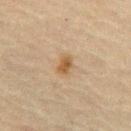Q: Is there a histopathology result?
A: imaged on a skin check; not biopsied
Q: Lesion size?
A: ~3 mm (longest diameter)
Q: How was the tile lit?
A: cross-polarized illumination
Q: What is the anatomic site?
A: the abdomen
Q: Who is the patient?
A: male, aged 68 to 72
Q: How was this image acquired?
A: total-body-photography crop, ~15 mm field of view